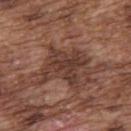workup=no biopsy performed (imaged during a skin exam); lesion diameter=about 5 mm; subject=male, aged 73–77; illumination=white-light illumination; imaging modality=~15 mm crop, total-body skin-cancer survey; anatomic site=the upper back.